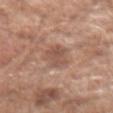The lesion is on the right forearm.
The lesion's longest dimension is about 4 mm.
Imaged with white-light lighting.
Automated tile analysis of the lesion measured an eccentricity of roughly 0.85. It also reported an automated nevus-likeness rating near 0 out of 100.
A roughly 15 mm field-of-view crop from a total-body skin photograph.
A male subject, aged 58 to 62.